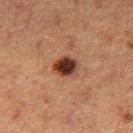The lesion was photographed on a routine skin check and not biopsied; there is no pathology result. A 15 mm close-up tile from a total-body photography series done for melanoma screening. The lesion-visualizer software estimated a footprint of about 6.5 mm² and two-axis asymmetry of about 0.15. The software also gave a peripheral color-asymmetry measure near 2. This is a cross-polarized tile. A female subject, in their 40s. The lesion is located on the right thigh.